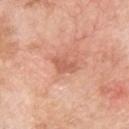A male patient, aged 58 to 62.
The lesion is on the upper back.
A close-up tile cropped from a whole-body skin photograph, about 15 mm across.